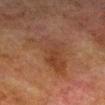Recorded during total-body skin imaging; not selected for excision or biopsy. A male patient, roughly 80 years of age. A close-up tile cropped from a whole-body skin photograph, about 15 mm across. The lesion is located on the right lower leg. The total-body-photography lesion software estimated a footprint of about 17 mm², a shape eccentricity near 0.8, and a shape-asymmetry score of about 0.4 (0 = symmetric). And it measured a classifier nevus-likeness of about 0/100 and a detector confidence of about 100 out of 100 that the crop contains a lesion.